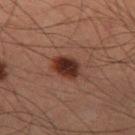The lesion is on the leg. A male subject, aged approximately 60. Automated tile analysis of the lesion measured a border-irregularity index near 1.5/10, a within-lesion color-variation index near 4/10, and peripheral color asymmetry of about 1.5. A 15 mm close-up extracted from a 3D total-body photography capture. Approximately 3.5 mm at its widest. This is a cross-polarized tile.